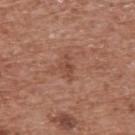Captured during whole-body skin photography for melanoma surveillance; the lesion was not biopsied. Approximately 3 mm at its widest. A male patient in their mid-70s. The lesion-visualizer software estimated an eccentricity of roughly 0.8 and a symmetry-axis asymmetry near 0.3. The analysis additionally found border irregularity of about 3.5 on a 0–10 scale, a within-lesion color-variation index near 2/10, and radial color variation of about 0.5. The analysis additionally found an automated nevus-likeness rating near 0 out of 100 and a detector confidence of about 100 out of 100 that the crop contains a lesion. The tile uses white-light illumination. A roughly 15 mm field-of-view crop from a total-body skin photograph. The lesion is on the back.